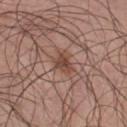Acquisition and patient details: A male subject approximately 30 years of age. A close-up tile cropped from a whole-body skin photograph, about 15 mm across. From the chest. Automated tile analysis of the lesion measured an area of roughly 4.5 mm², an eccentricity of roughly 0.65, and a shape-asymmetry score of about 0.35 (0 = symmetric). It also reported a border-irregularity index near 3/10. And it measured a lesion-detection confidence of about 100/100. The tile uses white-light illumination.